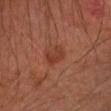biopsy status: catalogued during a skin exam; not biopsied | body site: the left forearm | patient: male, roughly 65 years of age | lesion diameter: ≈2.5 mm | image: 15 mm crop, total-body photography | tile lighting: cross-polarized | image-analysis metrics: an area of roughly 4.5 mm², an outline eccentricity of about 0.75 (0 = round, 1 = elongated), and two-axis asymmetry of about 0.3; a lesion color around L≈33 a*≈23 b*≈28 in CIELAB and roughly 7 lightness units darker than nearby skin; a classifier nevus-likeness of about 15/100 and a lesion-detection confidence of about 100/100.